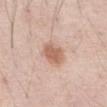Case summary:
- workup · no biopsy performed (imaged during a skin exam)